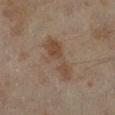Impression:
This lesion was catalogued during total-body skin photography and was not selected for biopsy.
Clinical summary:
A 15 mm close-up tile from a total-body photography series done for melanoma screening. Located on the left lower leg. The recorded lesion diameter is about 6 mm. Imaged with cross-polarized lighting. A female subject about 60 years old.